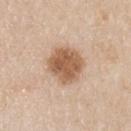A male subject approximately 35 years of age.
Approximately 4.5 mm at its widest.
Located on the upper back.
A close-up tile cropped from a whole-body skin photograph, about 15 mm across.